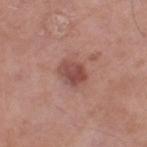<record>
<lighting>white-light</lighting>
<lesion_size>
  <long_diameter_mm_approx>3.0</long_diameter_mm_approx>
</lesion_size>
<automated_metrics>
  <vs_skin_darker_L>11.0</vs_skin_darker_L>
  <vs_skin_contrast_norm>8.0</vs_skin_contrast_norm>
  <lesion_detection_confidence_0_100>100</lesion_detection_confidence_0_100>
</automated_metrics>
<site>left thigh</site>
<image>
  <source>total-body photography crop</source>
  <field_of_view_mm>15</field_of_view_mm>
</image>
<patient>
  <sex>male</sex>
  <age_approx>55</age_approx>
</patient>
</record>